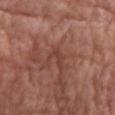biopsy status — no biopsy performed (imaged during a skin exam).